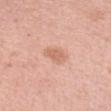Part of a total-body skin-imaging series; this lesion was reviewed on a skin check and was not flagged for biopsy.
Cropped from a total-body skin-imaging series; the visible field is about 15 mm.
Approximately 3 mm at its widest.
A female patient approximately 50 years of age.
Imaged with white-light lighting.
The lesion is on the left forearm.